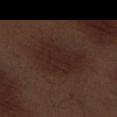biopsy status: catalogued during a skin exam; not biopsied | lesion diameter: ~6.5 mm (longest diameter) | image source: ~15 mm crop, total-body skin-cancer survey | site: the left thigh | tile lighting: white-light | TBP lesion metrics: a border-irregularity rating of about 3/10 | patient: male, aged 68–72.